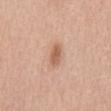Assessment:
Imaged during a routine full-body skin examination; the lesion was not biopsied and no histopathology is available.
Context:
Located on the abdomen. The tile uses white-light illumination. About 3 mm across. A close-up tile cropped from a whole-body skin photograph, about 15 mm across. The subject is a male aged approximately 75. The total-body-photography lesion software estimated an average lesion color of about L≈60 a*≈22 b*≈32 (CIELAB) and a lesion-to-skin contrast of about 7 (normalized; higher = more distinct). The software also gave border irregularity of about 1.5 on a 0–10 scale, internal color variation of about 2.5 on a 0–10 scale, and peripheral color asymmetry of about 1. The software also gave a classifier nevus-likeness of about 80/100 and a lesion-detection confidence of about 100/100.